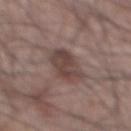Recorded during total-body skin imaging; not selected for excision or biopsy.
A male patient aged 63–67.
A lesion tile, about 15 mm wide, cut from a 3D total-body photograph.
This is a white-light tile.
The lesion is on the front of the torso.